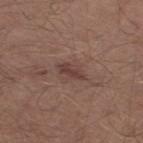Notes:
– workup · imaged on a skin check; not biopsied
– body site · the right thigh
– patient · male, in their mid- to late 60s
– imaging modality · 15 mm crop, total-body photography
– size · ≈3.5 mm
– image-analysis metrics · a mean CIELAB color near L≈40 a*≈19 b*≈21, a lesion–skin lightness drop of about 9, and a normalized lesion–skin contrast near 7.5; border irregularity of about 3 on a 0–10 scale, internal color variation of about 2 on a 0–10 scale, and peripheral color asymmetry of about 0.5; a nevus-likeness score of about 5/100 and lesion-presence confidence of about 95/100